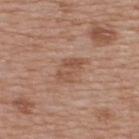Clinical impression: Imaged during a routine full-body skin examination; the lesion was not biopsied and no histopathology is available. Background: The total-body-photography lesion software estimated border irregularity of about 3.5 on a 0–10 scale, internal color variation of about 3 on a 0–10 scale, and a peripheral color-asymmetry measure near 1. The software also gave an automated nevus-likeness rating near 0 out of 100. The recorded lesion diameter is about 4 mm. The lesion is on the upper back. The subject is a male aged approximately 65. A 15 mm crop from a total-body photograph taken for skin-cancer surveillance. The tile uses white-light illumination.